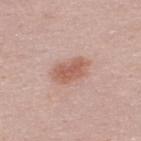{
  "biopsy_status": "not biopsied; imaged during a skin examination",
  "site": "upper back",
  "patient": {
    "sex": "male",
    "age_approx": 25
  },
  "image": {
    "source": "total-body photography crop",
    "field_of_view_mm": 15
  },
  "lighting": "white-light"
}